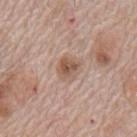notes: catalogued during a skin exam; not biopsied
lighting: white-light illumination
site: the mid back
lesion size: ~2.5 mm (longest diameter)
subject: male, aged around 70
acquisition: 15 mm crop, total-body photography
automated metrics: a border-irregularity index near 2/10, a color-variation rating of about 4/10, and radial color variation of about 1.5; lesion-presence confidence of about 100/100